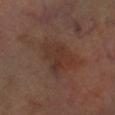Q: Was this lesion biopsied?
A: total-body-photography surveillance lesion; no biopsy
Q: Patient demographics?
A: female, approximately 60 years of age
Q: How was this image acquired?
A: ~15 mm tile from a whole-body skin photo
Q: What is the anatomic site?
A: the leg